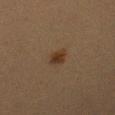Imaged during a routine full-body skin examination; the lesion was not biopsied and no histopathology is available.
Measured at roughly 2.5 mm in maximum diameter.
From the left upper arm.
An algorithmic analysis of the crop reported an area of roughly 3 mm², an eccentricity of roughly 0.8, and two-axis asymmetry of about 0.25. The analysis additionally found a lesion color around L≈27 a*≈14 b*≈24 in CIELAB and a lesion–skin lightness drop of about 7.
Cropped from a whole-body photographic skin survey; the tile spans about 15 mm.
The patient is a female aged approximately 40.